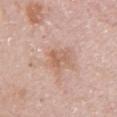image: total-body-photography crop, ~15 mm field of view; lighting: white-light; patient: female, aged 48 to 52; site: the left upper arm.